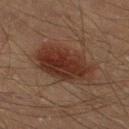• notes: imaged on a skin check; not biopsied
• diameter: ~7 mm (longest diameter)
• imaging modality: 15 mm crop, total-body photography
• TBP lesion metrics: a mean CIELAB color near L≈29 a*≈19 b*≈24 and roughly 10 lightness units darker than nearby skin
• patient: male, aged 38 to 42
• body site: the left lower leg
• tile lighting: cross-polarized